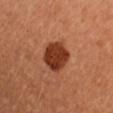Recorded during total-body skin imaging; not selected for excision or biopsy. A female subject, approximately 35 years of age. Captured under cross-polarized illumination. Cropped from a whole-body photographic skin survey; the tile spans about 15 mm. The total-body-photography lesion software estimated border irregularity of about 1.5 on a 0–10 scale and a peripheral color-asymmetry measure near 1. The software also gave a classifier nevus-likeness of about 100/100. On the right upper arm. The recorded lesion diameter is about 4 mm.